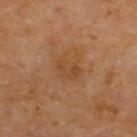Part of a total-body skin-imaging series; this lesion was reviewed on a skin check and was not flagged for biopsy. Approximately 3 mm at its widest. The lesion is located on the back. A male subject about 70 years old. The tile uses cross-polarized illumination. The lesion-visualizer software estimated a lesion area of about 6.5 mm², a shape eccentricity near 0.65, and two-axis asymmetry of about 0.25. It also reported a mean CIELAB color near L≈45 a*≈21 b*≈35, a lesion–skin lightness drop of about 5, and a normalized lesion–skin contrast near 5. The analysis additionally found a classifier nevus-likeness of about 0/100 and a lesion-detection confidence of about 100/100. A 15 mm crop from a total-body photograph taken for skin-cancer surveillance.